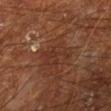biopsy status: no biopsy performed (imaged during a skin exam)
body site: the left lower leg
image source: 15 mm crop, total-body photography
patient: male, about 65 years old